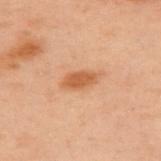workup: no biopsy performed (imaged during a skin exam); automated metrics: a mean CIELAB color near L≈49 a*≈22 b*≈34 and a lesion–skin lightness drop of about 9; size: ~4 mm (longest diameter); illumination: cross-polarized illumination; site: the upper back; imaging modality: 15 mm crop, total-body photography; patient: female, in their mid- to late 50s.